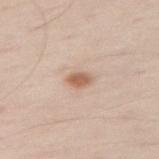Clinical impression: The lesion was tiled from a total-body skin photograph and was not biopsied. Clinical summary: The lesion-visualizer software estimated a mean CIELAB color near L≈61 a*≈19 b*≈31, about 12 CIELAB-L* units darker than the surrounding skin, and a normalized border contrast of about 8. A 15 mm close-up tile from a total-body photography series done for melanoma screening. A male subject in their 60s. From the right thigh. Measured at roughly 2.5 mm in maximum diameter. Imaged with white-light lighting.